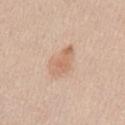workup — total-body-photography surveillance lesion; no biopsy
site — the right upper arm
image source — ~15 mm tile from a whole-body skin photo
subject — female, aged around 40